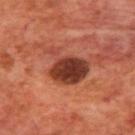| feature | finding |
|---|---|
| image | total-body-photography crop, ~15 mm field of view |
| anatomic site | the upper back |
| subject | male, approximately 70 years of age |
| size | ≈5.5 mm |